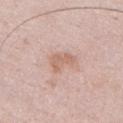Imaged during a routine full-body skin examination; the lesion was not biopsied and no histopathology is available. The subject is a male about 25 years old. Located on the chest. Approximately 3 mm at its widest. Cropped from a whole-body photographic skin survey; the tile spans about 15 mm. Automated tile analysis of the lesion measured a footprint of about 5 mm², an outline eccentricity of about 0.75 (0 = round, 1 = elongated), and two-axis asymmetry of about 0.5. And it measured an average lesion color of about L≈63 a*≈20 b*≈28 (CIELAB), a lesion–skin lightness drop of about 8, and a normalized lesion–skin contrast near 6. And it measured a border-irregularity rating of about 5.5/10 and a color-variation rating of about 1.5/10. The analysis additionally found a nevus-likeness score of about 0/100 and a detector confidence of about 100 out of 100 that the crop contains a lesion. Captured under white-light illumination.